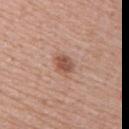The lesion was tiled from a total-body skin photograph and was not biopsied. The subject is a female in their 70s. Automated tile analysis of the lesion measured border irregularity of about 2.5 on a 0–10 scale, a within-lesion color-variation index near 2.5/10, and peripheral color asymmetry of about 1. The software also gave a lesion-detection confidence of about 100/100. The recorded lesion diameter is about 2.5 mm. The tile uses white-light illumination. A 15 mm crop from a total-body photograph taken for skin-cancer surveillance. On the upper back.